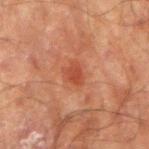Recorded during total-body skin imaging; not selected for excision or biopsy. The patient is a male aged 68–72. The recorded lesion diameter is about 2.5 mm. The lesion is on the right upper arm. A 15 mm crop from a total-body photograph taken for skin-cancer surveillance.